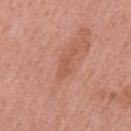Case summary:
• workup — no biopsy performed (imaged during a skin exam)
• tile lighting — white-light illumination
• size — ~2.5 mm (longest diameter)
• patient — male, in their mid- to late 50s
• body site — the mid back
• image — 15 mm crop, total-body photography